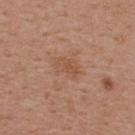follow-up = catalogued during a skin exam; not biopsied | lesion diameter = ≈3 mm | patient = male, in their mid- to late 50s | illumination = white-light illumination | site = the back | image source = total-body-photography crop, ~15 mm field of view.